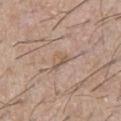biopsy status: catalogued during a skin exam; not biopsied
subject: male, aged 63 to 67
lesion size: ~2.5 mm (longest diameter)
automated metrics: a footprint of about 4 mm², an eccentricity of roughly 0.7, and a symmetry-axis asymmetry near 0.45; an automated nevus-likeness rating near 0 out of 100
illumination: white-light
image source: ~15 mm crop, total-body skin-cancer survey
body site: the chest